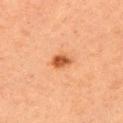Part of a total-body skin-imaging series; this lesion was reviewed on a skin check and was not flagged for biopsy. On the left upper arm. Imaged with cross-polarized lighting. The subject is a female aged approximately 60. A 15 mm close-up extracted from a 3D total-body photography capture. The lesion's longest dimension is about 2.5 mm.